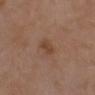Context:
A roughly 15 mm field-of-view crop from a total-body skin photograph. About 2.5 mm across. The subject is a female approximately 40 years of age. The tile uses white-light illumination. The lesion is on the chest.